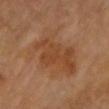Clinical impression: The lesion was tiled from a total-body skin photograph and was not biopsied. Image and clinical context: The subject is a female roughly 70 years of age. A close-up tile cropped from a whole-body skin photograph, about 15 mm across. The lesion is on the chest. The total-body-photography lesion software estimated a footprint of about 29 mm², an eccentricity of roughly 0.75, and two-axis asymmetry of about 0.35. The analysis additionally found a lesion color around L≈43 a*≈20 b*≈33 in CIELAB, about 6 CIELAB-L* units darker than the surrounding skin, and a normalized border contrast of about 6. The software also gave border irregularity of about 5.5 on a 0–10 scale, a color-variation rating of about 3.5/10, and a peripheral color-asymmetry measure near 1.5.